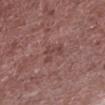Notes:
- workup · imaged on a skin check; not biopsied
- image · ~15 mm tile from a whole-body skin photo
- body site · the left lower leg
- subject · male, aged around 65
- tile lighting · white-light illumination